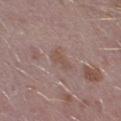Impression: Imaged during a routine full-body skin examination; the lesion was not biopsied and no histopathology is available. Context: A male subject roughly 40 years of age. An algorithmic analysis of the crop reported an area of roughly 3 mm² and a symmetry-axis asymmetry near 0.45. The analysis additionally found a border-irregularity index near 4.5/10, a within-lesion color-variation index near 0.5/10, and radial color variation of about 0. From the left lower leg. Approximately 2.5 mm at its widest. A lesion tile, about 15 mm wide, cut from a 3D total-body photograph.